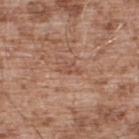Assessment: Part of a total-body skin-imaging series; this lesion was reviewed on a skin check and was not flagged for biopsy. Image and clinical context: The tile uses white-light illumination. The lesion's longest dimension is about 2.5 mm. Automated image analysis of the tile measured an area of roughly 2.5 mm², an outline eccentricity of about 0.85 (0 = round, 1 = elongated), and a shape-asymmetry score of about 0.35 (0 = symmetric). And it measured an average lesion color of about L≈51 a*≈22 b*≈29 (CIELAB), about 7 CIELAB-L* units darker than the surrounding skin, and a normalized border contrast of about 5. The analysis additionally found a border-irregularity index near 4/10 and radial color variation of about 0. The subject is a male aged 53 to 57. Located on the upper back. A region of skin cropped from a whole-body photographic capture, roughly 15 mm wide.